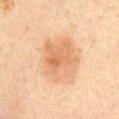• notes — catalogued during a skin exam; not biopsied